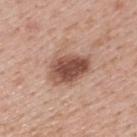{"biopsy_status": "not biopsied; imaged during a skin examination", "patient": {"sex": "male", "age_approx": 40}, "lesion_size": {"long_diameter_mm_approx": 4.5}, "image": {"source": "total-body photography crop", "field_of_view_mm": 15}, "site": "upper back"}